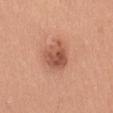Q: Was this lesion biopsied?
A: catalogued during a skin exam; not biopsied
Q: Where on the body is the lesion?
A: the back
Q: How large is the lesion?
A: about 4 mm
Q: Patient demographics?
A: female, in their mid-30s
Q: What is the imaging modality?
A: ~15 mm tile from a whole-body skin photo